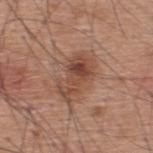{
  "biopsy_status": "not biopsied; imaged during a skin examination",
  "patient": {
    "sex": "male",
    "age_approx": 60
  },
  "lesion_size": {
    "long_diameter_mm_approx": 5.0
  },
  "image": {
    "source": "total-body photography crop",
    "field_of_view_mm": 15
  },
  "site": "upper back"
}